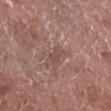Findings:
* workup — total-body-photography surveillance lesion; no biopsy
* image source — ~15 mm crop, total-body skin-cancer survey
* automated lesion analysis — an average lesion color of about L≈48 a*≈19 b*≈22 (CIELAB), roughly 6 lightness units darker than nearby skin, and a normalized border contrast of about 5; a within-lesion color-variation index near 0.5/10 and peripheral color asymmetry of about 0; an automated nevus-likeness rating near 0 out of 100 and a detector confidence of about 70 out of 100 that the crop contains a lesion
* patient — male, aged 73–77
* body site — the right lower leg
* size — ≈2.5 mm
* illumination — white-light illumination